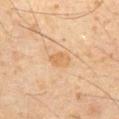Clinical summary:
About 2.5 mm across. Automated image analysis of the tile measured a lesion color around L≈54 a*≈17 b*≈35 in CIELAB and about 6 CIELAB-L* units darker than the surrounding skin. A region of skin cropped from a whole-body photographic capture, roughly 15 mm wide. On the front of the torso. A male patient, aged around 60. Imaged with cross-polarized lighting.